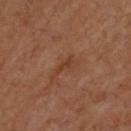Findings:
– notes · no biopsy performed (imaged during a skin exam)
– acquisition · ~15 mm tile from a whole-body skin photo
– anatomic site · the upper back
– subject · aged 63 to 67
– image-analysis metrics · a footprint of about 2 mm²; a nevus-likeness score of about 0/100 and a detector confidence of about 100 out of 100 that the crop contains a lesion
– lighting · cross-polarized illumination
– lesion diameter · ≈2.5 mm